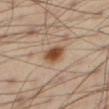| field | value |
|---|---|
| workup | no biopsy performed (imaged during a skin exam) |
| subject | male, about 55 years old |
| location | the right thigh |
| acquisition | total-body-photography crop, ~15 mm field of view |
| tile lighting | cross-polarized |